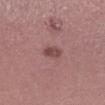| feature | finding |
|---|---|
| follow-up | total-body-photography surveillance lesion; no biopsy |
| anatomic site | the left lower leg |
| patient | male, aged 43–47 |
| acquisition | 15 mm crop, total-body photography |